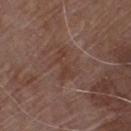Q: Is there a histopathology result?
A: total-body-photography surveillance lesion; no biopsy
Q: What is the anatomic site?
A: the front of the torso
Q: What are the patient's age and sex?
A: male, in their 80s
Q: How large is the lesion?
A: ~4 mm (longest diameter)
Q: Automated lesion metrics?
A: a lesion area of about 6 mm²; an average lesion color of about L≈39 a*≈18 b*≈24 (CIELAB), about 5 CIELAB-L* units darker than the surrounding skin, and a normalized lesion–skin contrast near 5; a border-irregularity index near 7.5/10, a within-lesion color-variation index near 3/10, and radial color variation of about 1; a classifier nevus-likeness of about 0/100 and a detector confidence of about 100 out of 100 that the crop contains a lesion
Q: What kind of image is this?
A: 15 mm crop, total-body photography